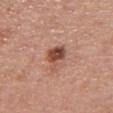  site: chest
  automated_metrics:
    area_mm2_approx: 6.0
    shape_asymmetry: 0.4
    border_irregularity_0_10: 4.0
    peripheral_color_asymmetry: 2.0
    nevus_likeness_0_100: 80
    lesion_detection_confidence_0_100: 100
  patient:
    sex: female
    age_approx: 55
  image:
    source: total-body photography crop
    field_of_view_mm: 15